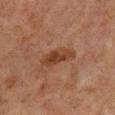follow-up: no biopsy performed (imaged during a skin exam) | size: ≈4 mm | patient: male, roughly 60 years of age | body site: the back | tile lighting: cross-polarized | image source: ~15 mm crop, total-body skin-cancer survey.